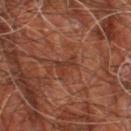biopsy status: imaged on a skin check; not biopsied
tile lighting: cross-polarized
subject: male, aged around 60
anatomic site: the right leg
lesion diameter: about 2.5 mm
acquisition: 15 mm crop, total-body photography